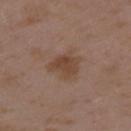notes: total-body-photography surveillance lesion; no biopsy | subject: female, in their mid-30s | imaging modality: ~15 mm crop, total-body skin-cancer survey | image-analysis metrics: a classifier nevus-likeness of about 5/100 and a lesion-detection confidence of about 100/100 | body site: the left upper arm.